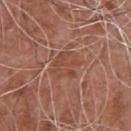Findings:
- notes: imaged on a skin check; not biopsied
- TBP lesion metrics: a lesion color around L≈47 a*≈23 b*≈29 in CIELAB, roughly 6 lightness units darker than nearby skin, and a normalized border contrast of about 5; a border-irregularity rating of about 6/10, a within-lesion color-variation index near 3/10, and a peripheral color-asymmetry measure near 1
- patient: male, in their mid- to late 70s
- imaging modality: total-body-photography crop, ~15 mm field of view
- body site: the chest
- lesion size: ~3.5 mm (longest diameter)